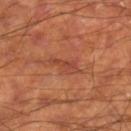biopsy_status: not biopsied; imaged during a skin examination
automated_metrics:
  border_irregularity_0_10: 5.0
  color_variation_0_10: 1.0
lesion_size:
  long_diameter_mm_approx: 3.5
image:
  source: total-body photography crop
  field_of_view_mm: 15
patient:
  sex: male
  age_approx: 60
site: right lower leg
lighting: cross-polarized